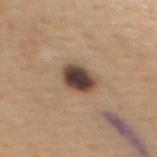Clinical summary:
Located on the upper back. A region of skin cropped from a whole-body photographic capture, roughly 15 mm wide. A female subject, roughly 45 years of age.
Conclusion:
Histopathology of the biopsied lesion showed a dysplastic (Clark) nevus, classified as a benign skin lesion.